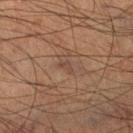Imaged during a routine full-body skin examination; the lesion was not biopsied and no histopathology is available. Imaged with cross-polarized lighting. A 15 mm close-up extracted from a 3D total-body photography capture. On the right lower leg. A male subject, aged 58 to 62.